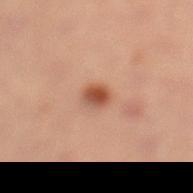Q: Was this lesion biopsied?
A: no biopsy performed (imaged during a skin exam)